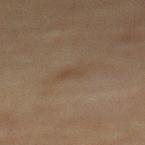– body site — the mid back
– tile lighting — cross-polarized
– imaging modality — ~15 mm crop, total-body skin-cancer survey
– automated lesion analysis — an average lesion color of about L≈38 a*≈12 b*≈25 (CIELAB) and a normalized border contrast of about 4.5
– subject — female, in their 70s
– lesion diameter — ~2.5 mm (longest diameter)